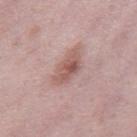Part of a total-body skin-imaging series; this lesion was reviewed on a skin check and was not flagged for biopsy. The lesion's longest dimension is about 4.5 mm. The tile uses white-light illumination. Cropped from a total-body skin-imaging series; the visible field is about 15 mm. A female subject, about 40 years old. From the right thigh.